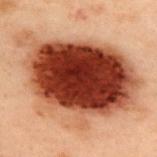| feature | finding |
|---|---|
| patient | male, aged approximately 55 |
| size | ~13.5 mm (longest diameter) |
| site | the upper back |
| image source | 15 mm crop, total-body photography |